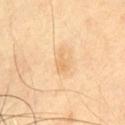Captured during whole-body skin photography for melanoma surveillance; the lesion was not biopsied.
The subject is a male aged around 40.
Captured under cross-polarized illumination.
Cropped from a whole-body photographic skin survey; the tile spans about 15 mm.
Located on the chest.